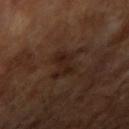Image and clinical context: Cropped from a total-body skin-imaging series; the visible field is about 15 mm. The lesion is on the right upper arm. A male patient roughly 65 years of age.